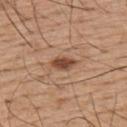Clinical impression: Part of a total-body skin-imaging series; this lesion was reviewed on a skin check and was not flagged for biopsy. Acquisition and patient details: Measured at roughly 3 mm in maximum diameter. The patient is a male roughly 55 years of age. A 15 mm crop from a total-body photograph taken for skin-cancer surveillance. The tile uses white-light illumination. From the upper back. An algorithmic analysis of the crop reported an average lesion color of about L≈47 a*≈21 b*≈31 (CIELAB), roughly 14 lightness units darker than nearby skin, and a normalized border contrast of about 9.5. And it measured a border-irregularity index near 2/10 and peripheral color asymmetry of about 1. And it measured a classifier nevus-likeness of about 95/100 and lesion-presence confidence of about 100/100.